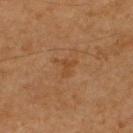Q: Was a biopsy performed?
A: catalogued during a skin exam; not biopsied
Q: How large is the lesion?
A: ≈2.5 mm
Q: Lesion location?
A: the upper back
Q: What is the imaging modality?
A: 15 mm crop, total-body photography
Q: Automated lesion metrics?
A: an average lesion color of about L≈37 a*≈18 b*≈31 (CIELAB), roughly 4 lightness units darker than nearby skin, and a normalized border contrast of about 5; a border-irregularity index near 6.5/10, internal color variation of about 0.5 on a 0–10 scale, and a peripheral color-asymmetry measure near 0; a nevus-likeness score of about 0/100 and a lesion-detection confidence of about 100/100
Q: Who is the patient?
A: male, in their mid-60s